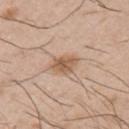Part of a total-body skin-imaging series; this lesion was reviewed on a skin check and was not flagged for biopsy.
This image is a 15 mm lesion crop taken from a total-body photograph.
A male patient, aged approximately 50.
From the left upper arm.
The lesion's longest dimension is about 3.5 mm.
Automated image analysis of the tile measured a color-variation rating of about 3.5/10 and radial color variation of about 1.5.